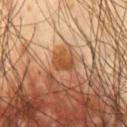Recorded during total-body skin imaging; not selected for excision or biopsy. The lesion is on the chest. A male patient, in their mid- to late 50s. Cropped from a total-body skin-imaging series; the visible field is about 15 mm.